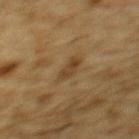workup: total-body-photography surveillance lesion; no biopsy
imaging modality: 15 mm crop, total-body photography
patient: male, aged around 85 or older
diameter: about 2.5 mm
location: the upper back
lighting: cross-polarized